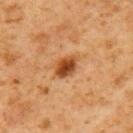Clinical impression:
Captured during whole-body skin photography for melanoma surveillance; the lesion was not biopsied.
Context:
This image is a 15 mm lesion crop taken from a total-body photograph. The subject is a male roughly 60 years of age. The lesion is located on the left upper arm. The lesion-visualizer software estimated a lesion–skin lightness drop of about 13 and a normalized lesion–skin contrast near 10.5. Approximately 3.5 mm at its widest.